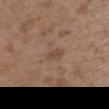Notes:
• workup · imaged on a skin check; not biopsied
• subject · female, aged 33 to 37
• image · ~15 mm crop, total-body skin-cancer survey
• body site · the upper back
• tile lighting · white-light illumination
• automated lesion analysis · a footprint of about 2.5 mm², a shape eccentricity near 0.9, and two-axis asymmetry of about 0.3; a border-irregularity rating of about 3/10 and radial color variation of about 0